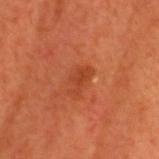Acquisition and patient details:
The lesion is on the head or neck. The subject is a male aged around 60. A close-up tile cropped from a whole-body skin photograph, about 15 mm across.